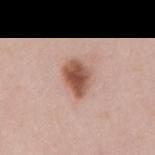Q: Was a biopsy performed?
A: catalogued during a skin exam; not biopsied
Q: What kind of image is this?
A: ~15 mm crop, total-body skin-cancer survey
Q: What did automated image analysis measure?
A: a symmetry-axis asymmetry near 0.2; a border-irregularity index near 2/10 and radial color variation of about 1.5; a classifier nevus-likeness of about 100/100
Q: How large is the lesion?
A: ~4 mm (longest diameter)
Q: Illumination type?
A: white-light
Q: Who is the patient?
A: female, aged 38 to 42
Q: Lesion location?
A: the mid back